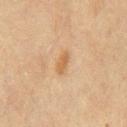{
  "biopsy_status": "not biopsied; imaged during a skin examination",
  "patient": {
    "sex": "male",
    "age_approx": 70
  },
  "site": "chest",
  "lighting": "cross-polarized",
  "image": {
    "source": "total-body photography crop",
    "field_of_view_mm": 15
  },
  "automated_metrics": {
    "area_mm2_approx": 3.5,
    "eccentricity": 0.9,
    "cielab_L": 50,
    "cielab_a": 16,
    "cielab_b": 33,
    "vs_skin_darker_L": 7.0,
    "vs_skin_contrast_norm": 6.5
  }
}